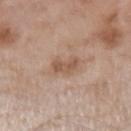| key | value |
|---|---|
| notes | no biopsy performed (imaged during a skin exam) |
| acquisition | 15 mm crop, total-body photography |
| diameter | ≈2.5 mm |
| image-analysis metrics | a lesion-to-skin contrast of about 6.5 (normalized; higher = more distinct) |
| subject | male, aged approximately 55 |
| illumination | white-light |
| location | the left upper arm |